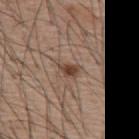Q: Was this lesion biopsied?
A: total-body-photography surveillance lesion; no biopsy
Q: How was the tile lit?
A: white-light
Q: How large is the lesion?
A: ~2.5 mm (longest diameter)
Q: Who is the patient?
A: male, aged 53 to 57
Q: Automated lesion metrics?
A: an area of roughly 3.5 mm², an eccentricity of roughly 0.75, and a symmetry-axis asymmetry near 0.35; a lesion-to-skin contrast of about 9 (normalized; higher = more distinct)
Q: How was this image acquired?
A: 15 mm crop, total-body photography
Q: What is the anatomic site?
A: the upper back